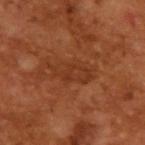Clinical impression: Recorded during total-body skin imaging; not selected for excision or biopsy. Background: Longest diameter approximately 5 mm. This is a cross-polarized tile. This image is a 15 mm lesion crop taken from a total-body photograph. A male subject, in their mid-60s.